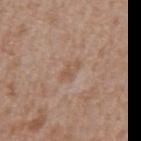Captured during whole-body skin photography for melanoma surveillance; the lesion was not biopsied.
A male subject, about 65 years old.
On the mid back.
A roughly 15 mm field-of-view crop from a total-body skin photograph.